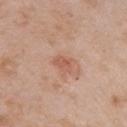| key | value |
|---|---|
| follow-up | no biopsy performed (imaged during a skin exam) |
| site | the left upper arm |
| patient | female, aged 68 to 72 |
| illumination | white-light illumination |
| diameter | ≈2.5 mm |
| imaging modality | ~15 mm crop, total-body skin-cancer survey |